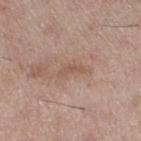The lesion was tiled from a total-body skin photograph and was not biopsied.
An algorithmic analysis of the crop reported an average lesion color of about L≈54 a*≈18 b*≈28 (CIELAB) and a normalized lesion–skin contrast near 5.5. It also reported a border-irregularity rating of about 6/10 and radial color variation of about 0.
Captured under white-light illumination.
On the right thigh.
A region of skin cropped from a whole-body photographic capture, roughly 15 mm wide.
The recorded lesion diameter is about 3 mm.
The subject is a male aged 48–52.